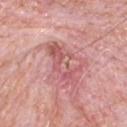Clinical impression: The lesion was photographed on a routine skin check and not biopsied; there is no pathology result. Image and clinical context: A male patient aged 78 to 82. A region of skin cropped from a whole-body photographic capture, roughly 15 mm wide. From the chest. The lesion-visualizer software estimated an area of roughly 10 mm², a shape eccentricity near 0.9, and two-axis asymmetry of about 0.4. The analysis additionally found a mean CIELAB color near L≈57 a*≈29 b*≈22, about 9 CIELAB-L* units darker than the surrounding skin, and a lesion-to-skin contrast of about 6 (normalized; higher = more distinct).